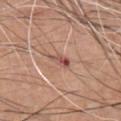Assessment: Imaged during a routine full-body skin examination; the lesion was not biopsied and no histopathology is available. Image and clinical context: A 15 mm crop from a total-body photograph taken for skin-cancer surveillance. A male patient approximately 60 years of age. The lesion is on the chest. Captured under white-light illumination. The recorded lesion diameter is about 3 mm.